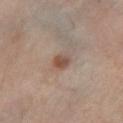biopsy status: catalogued during a skin exam; not biopsied
subject: male, aged around 70
anatomic site: the left lower leg
acquisition: 15 mm crop, total-body photography